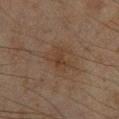This lesion was catalogued during total-body skin photography and was not selected for biopsy. The recorded lesion diameter is about 4.5 mm. An algorithmic analysis of the crop reported an eccentricity of roughly 0.8 and a symmetry-axis asymmetry near 0.45. And it measured a classifier nevus-likeness of about 0/100 and a lesion-detection confidence of about 100/100. The tile uses cross-polarized illumination. The subject is a male aged around 45. The lesion is located on the right lower leg. A 15 mm close-up tile from a total-body photography series done for melanoma screening.